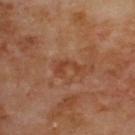| field | value |
|---|---|
| workup | catalogued during a skin exam; not biopsied |
| lighting | cross-polarized |
| subject | male, about 70 years old |
| site | the upper back |
| automated metrics | a border-irregularity index near 7/10, a within-lesion color-variation index near 0/10, and peripheral color asymmetry of about 0 |
| lesion diameter | about 3 mm |
| image source | ~15 mm tile from a whole-body skin photo |